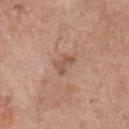The lesion was photographed on a routine skin check and not biopsied; there is no pathology result.
From the chest.
Measured at roughly 3 mm in maximum diameter.
A 15 mm close-up tile from a total-body photography series done for melanoma screening.
A male patient aged approximately 70.
Captured under white-light illumination.